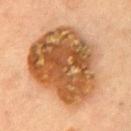Clinical summary:
Located on the front of the torso. The patient is a female aged around 60. A region of skin cropped from a whole-body photographic capture, roughly 15 mm wide. The recorded lesion diameter is about 9.5 mm. The tile uses cross-polarized illumination.